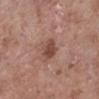About 3 mm across. A lesion tile, about 15 mm wide, cut from a 3D total-body photograph. The subject is a female roughly 75 years of age. The total-body-photography lesion software estimated a footprint of about 5.5 mm², a shape eccentricity near 0.55, and two-axis asymmetry of about 0.15. And it measured border irregularity of about 1.5 on a 0–10 scale, internal color variation of about 2.5 on a 0–10 scale, and a peripheral color-asymmetry measure near 0.5. It also reported an automated nevus-likeness rating near 30 out of 100. The tile uses white-light illumination. The lesion is on the leg.